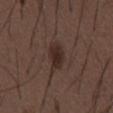follow-up — total-body-photography surveillance lesion; no biopsy
subject — male, about 50 years old
image — total-body-photography crop, ~15 mm field of view
lesion diameter — ~4 mm (longest diameter)
location — the abdomen